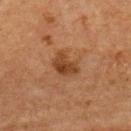Impression:
Recorded during total-body skin imaging; not selected for excision or biopsy.
Clinical summary:
On the back. Longest diameter approximately 3.5 mm. A region of skin cropped from a whole-body photographic capture, roughly 15 mm wide. This is a cross-polarized tile. A female patient, aged 58 to 62.